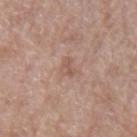Imaged during a routine full-body skin examination; the lesion was not biopsied and no histopathology is available. This image is a 15 mm lesion crop taken from a total-body photograph. The lesion is located on the left upper arm. Longest diameter approximately 2.5 mm. A male patient, aged 68 to 72.